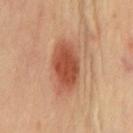notes = no biopsy performed (imaged during a skin exam); site = the chest; image source = total-body-photography crop, ~15 mm field of view; tile lighting = cross-polarized illumination.